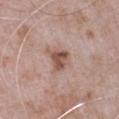The lesion was photographed on a routine skin check and not biopsied; there is no pathology result.
A lesion tile, about 15 mm wide, cut from a 3D total-body photograph.
A male patient, roughly 65 years of age.
On the right upper arm.